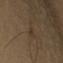Impression:
Recorded during total-body skin imaging; not selected for excision or biopsy.
Background:
The subject is a male about 60 years old. On the right arm. Cropped from a total-body skin-imaging series; the visible field is about 15 mm.